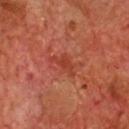follow-up: total-body-photography surveillance lesion; no biopsy
lesion diameter: ~3.5 mm (longest diameter)
acquisition: total-body-photography crop, ~15 mm field of view
site: the chest
subject: male, in their 70s
illumination: cross-polarized illumination
TBP lesion metrics: a footprint of about 4.5 mm², an outline eccentricity of about 0.85 (0 = round, 1 = elongated), and two-axis asymmetry of about 0.6; border irregularity of about 6.5 on a 0–10 scale and radial color variation of about 0.5; lesion-presence confidence of about 95/100